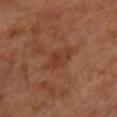biopsy_status: not biopsied; imaged during a skin examination
automated_metrics:
  area_mm2_approx: 7.0
  eccentricity: 0.9
  shape_asymmetry: 0.2
  cielab_L: 37
  cielab_a: 23
  cielab_b: 31
  vs_skin_darker_L: 6.0
  vs_skin_contrast_norm: 5.5
  color_variation_0_10: 2.0
  peripheral_color_asymmetry: 0.5
lighting: cross-polarized
image:
  source: total-body photography crop
  field_of_view_mm: 15
site: chest
patient:
  sex: male
  age_approx: 65